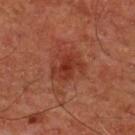This lesion was catalogued during total-body skin photography and was not selected for biopsy. The lesion's longest dimension is about 4 mm. The lesion is located on the chest. Captured under cross-polarized illumination. A 15 mm close-up extracted from a 3D total-body photography capture. A male patient, aged 58 to 62.